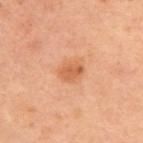Captured during whole-body skin photography for melanoma surveillance; the lesion was not biopsied.
The recorded lesion diameter is about 2.5 mm.
The lesion is on the left upper arm.
Imaged with cross-polarized lighting.
Automated tile analysis of the lesion measured a mean CIELAB color near L≈50 a*≈23 b*≈34, a lesion–skin lightness drop of about 8, and a lesion-to-skin contrast of about 6.5 (normalized; higher = more distinct). The software also gave a classifier nevus-likeness of about 70/100 and a lesion-detection confidence of about 100/100.
The subject is a female aged approximately 55.
A 15 mm crop from a total-body photograph taken for skin-cancer surveillance.